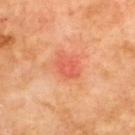Q: Is there a histopathology result?
A: catalogued during a skin exam; not biopsied
Q: What kind of image is this?
A: ~15 mm tile from a whole-body skin photo
Q: What is the lesion's diameter?
A: ≈3 mm
Q: Lesion location?
A: the upper back
Q: How was the tile lit?
A: cross-polarized illumination
Q: What did automated image analysis measure?
A: a shape eccentricity near 0.65 and a symmetry-axis asymmetry near 0.2; an average lesion color of about L≈59 a*≈36 b*≈37 (CIELAB) and a lesion-to-skin contrast of about 5 (normalized; higher = more distinct); a nevus-likeness score of about 0/100 and a lesion-detection confidence of about 100/100
Q: What are the patient's age and sex?
A: male, aged approximately 70